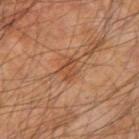{"biopsy_status": "not biopsied; imaged during a skin examination", "patient": {"sex": "male", "age_approx": 65}, "lighting": "cross-polarized", "image": {"source": "total-body photography crop", "field_of_view_mm": 15}, "site": "right upper arm"}